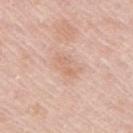biopsy status: imaged on a skin check; not biopsied | subject: female, aged approximately 65 | body site: the right upper arm | image-analysis metrics: a mean CIELAB color near L≈67 a*≈20 b*≈31, a lesion–skin lightness drop of about 6, and a lesion-to-skin contrast of about 5 (normalized; higher = more distinct); a border-irregularity rating of about 3.5/10, internal color variation of about 2 on a 0–10 scale, and peripheral color asymmetry of about 0.5 | size: ≈3 mm | acquisition: total-body-photography crop, ~15 mm field of view | tile lighting: white-light.